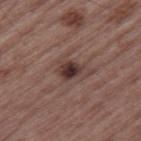biopsy status: no biopsy performed (imaged during a skin exam); anatomic site: the right thigh; patient: male, aged around 65; image: 15 mm crop, total-body photography.